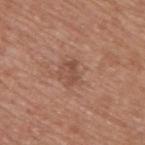The lesion was photographed on a routine skin check and not biopsied; there is no pathology result. The lesion is located on the back. Imaged with white-light lighting. A male patient, aged 63 to 67. A 15 mm crop from a total-body photograph taken for skin-cancer surveillance. Longest diameter approximately 2.5 mm.